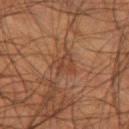<tbp_lesion>
<biopsy_status>not biopsied; imaged during a skin examination</biopsy_status>
<patient>
  <sex>male</sex>
  <age_approx>55</age_approx>
</patient>
<site>left thigh</site>
<image>
  <source>total-body photography crop</source>
  <field_of_view_mm>15</field_of_view_mm>
</image>
</tbp_lesion>